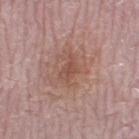workup: no biopsy performed (imaged during a skin exam)
TBP lesion metrics: a lesion color around L≈50 a*≈22 b*≈26 in CIELAB, a lesion–skin lightness drop of about 8, and a lesion-to-skin contrast of about 6 (normalized; higher = more distinct)
subject: male, aged around 65
imaging modality: ~15 mm crop, total-body skin-cancer survey
lesion diameter: ≈3 mm
tile lighting: white-light
anatomic site: the leg